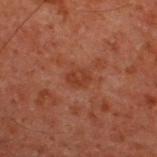<case>
  <biopsy_status>not biopsied; imaged during a skin examination</biopsy_status>
  <patient>
    <sex>male</sex>
    <age_approx>60</age_approx>
  </patient>
  <site>upper back</site>
  <image>
    <source>total-body photography crop</source>
    <field_of_view_mm>15</field_of_view_mm>
  </image>
  <lesion_size>
    <long_diameter_mm_approx>2.5</long_diameter_mm_approx>
  </lesion_size>
  <automated_metrics>
    <area_mm2_approx>4.0</area_mm2_approx>
    <eccentricity>0.7</eccentricity>
    <shape_asymmetry>0.25</shape_asymmetry>
    <border_irregularity_0_10>2.0</border_irregularity_0_10>
  </automated_metrics>
  <lighting>cross-polarized</lighting>
</case>